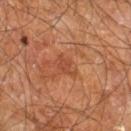No biopsy was performed on this lesion — it was imaged during a full skin examination and was not determined to be concerning.
Captured under cross-polarized illumination.
An algorithmic analysis of the crop reported a lesion color around L≈45 a*≈27 b*≈34 in CIELAB and a normalized border contrast of about 5. And it measured a border-irregularity index near 5/10, a color-variation rating of about 1/10, and radial color variation of about 0.5. The software also gave a classifier nevus-likeness of about 0/100.
Cropped from a total-body skin-imaging series; the visible field is about 15 mm.
Located on the right leg.
A male patient, approximately 60 years of age.
Longest diameter approximately 2.5 mm.